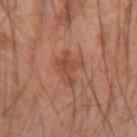Context: Captured under cross-polarized illumination. Approximately 3.5 mm at its widest. Cropped from a total-body skin-imaging series; the visible field is about 15 mm. Located on the left forearm. A male patient in their 60s. Automated tile analysis of the lesion measured a lesion color around L≈45 a*≈23 b*≈29 in CIELAB, roughly 7 lightness units darker than nearby skin, and a normalized lesion–skin contrast near 6. It also reported a nevus-likeness score of about 0/100 and lesion-presence confidence of about 100/100.